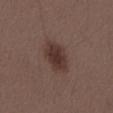workup = catalogued during a skin exam; not biopsied